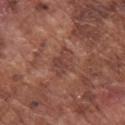biopsy status: imaged on a skin check; not biopsied | automated lesion analysis: an area of roughly 6.5 mm² and a shape-asymmetry score of about 0.35 (0 = symmetric); a lesion color around L≈42 a*≈22 b*≈25 in CIELAB, a lesion–skin lightness drop of about 7, and a normalized lesion–skin contrast near 5.5; a border-irregularity index near 3.5/10, a color-variation rating of about 2.5/10, and peripheral color asymmetry of about 1 | lesion size: ~3.5 mm (longest diameter) | location: the arm | image source: total-body-photography crop, ~15 mm field of view | subject: male, in their mid-70s.